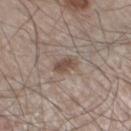{"lighting": "white-light", "lesion_size": {"long_diameter_mm_approx": 3.0}, "patient": {"sex": "male", "age_approx": 60}, "site": "left lower leg", "image": {"source": "total-body photography crop", "field_of_view_mm": 15}}